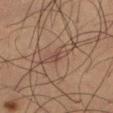workup = imaged on a skin check; not biopsied | body site = the right thigh | acquisition = 15 mm crop, total-body photography | tile lighting = cross-polarized illumination | subject = male, aged around 50 | size = about 3 mm | TBP lesion metrics = a lesion area of about 3 mm², an eccentricity of roughly 0.9, and two-axis asymmetry of about 0.2; border irregularity of about 2.5 on a 0–10 scale and internal color variation of about 1 on a 0–10 scale; a nevus-likeness score of about 0/100 and a detector confidence of about 75 out of 100 that the crop contains a lesion.